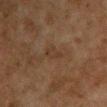Notes:
* workup — catalogued during a skin exam; not biopsied
* acquisition — ~15 mm crop, total-body skin-cancer survey
* size — about 3 mm
* image-analysis metrics — a lesion color around L≈32 a*≈15 b*≈26 in CIELAB, a lesion–skin lightness drop of about 4, and a lesion-to-skin contrast of about 5 (normalized; higher = more distinct); a classifier nevus-likeness of about 0/100 and a lesion-detection confidence of about 100/100
* body site — the chest
* patient — female, aged 58 to 62
* lighting — cross-polarized